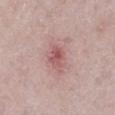  biopsy_status: not biopsied; imaged during a skin examination
  automated_metrics:
    vs_skin_contrast_norm: 7.0
    border_irregularity_0_10: 3.5
    color_variation_0_10: 5.0
    peripheral_color_asymmetry: 1.5
  patient:
    sex: male
    age_approx: 60
  image:
    source: total-body photography crop
    field_of_view_mm: 15
  lighting: white-light
  site: left lower leg
  lesion_size:
    long_diameter_mm_approx: 3.0